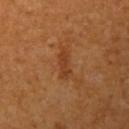follow-up: total-body-photography surveillance lesion; no biopsy
anatomic site: the arm
image: ~15 mm tile from a whole-body skin photo
patient: female